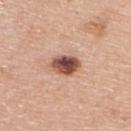Assessment: Captured during whole-body skin photography for melanoma surveillance; the lesion was not biopsied. Context: Imaged with white-light lighting. The recorded lesion diameter is about 3.5 mm. A female patient, aged 58–62. The lesion-visualizer software estimated about 19 CIELAB-L* units darker than the surrounding skin and a lesion-to-skin contrast of about 12.5 (normalized; higher = more distinct). A 15 mm close-up tile from a total-body photography series done for melanoma screening. Located on the right upper arm.